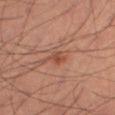biopsy status: catalogued during a skin exam; not biopsied | image: ~15 mm tile from a whole-body skin photo | location: the right thigh | subject: male, about 60 years old | automated lesion analysis: an area of roughly 3.5 mm² and an outline eccentricity of about 0.7 (0 = round, 1 = elongated); a lesion color around L≈44 a*≈22 b*≈29 in CIELAB, about 7 CIELAB-L* units darker than the surrounding skin, and a normalized border contrast of about 6; border irregularity of about 2 on a 0–10 scale, internal color variation of about 2.5 on a 0–10 scale, and radial color variation of about 0.5 | lighting: cross-polarized | lesion diameter: ~2.5 mm (longest diameter).